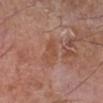Cropped from a total-body skin-imaging series; the visible field is about 15 mm.
On the right lower leg.
A male patient, approximately 60 years of age.